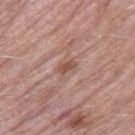Clinical impression: No biopsy was performed on this lesion — it was imaged during a full skin examination and was not determined to be concerning. Acquisition and patient details: A 15 mm crop from a total-body photograph taken for skin-cancer surveillance. From the right thigh. A female subject, in their 70s. Captured under white-light illumination. The recorded lesion diameter is about 2.5 mm. The lesion-visualizer software estimated a footprint of about 3 mm² and a shape eccentricity near 0.8. It also reported border irregularity of about 2 on a 0–10 scale and a peripheral color-asymmetry measure near 1.